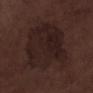No biopsy was performed on this lesion — it was imaged during a full skin examination and was not determined to be concerning.
The tile uses white-light illumination.
Longest diameter approximately 8.5 mm.
A male patient, about 70 years old.
This image is a 15 mm lesion crop taken from a total-body photograph.
The lesion is on the left lower leg.